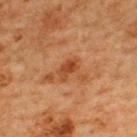Notes:
– workup — catalogued during a skin exam; not biopsied
– diameter — ≈3 mm
– illumination — cross-polarized
– patient — male, aged 63–67
– site — the upper back
– image — ~15 mm tile from a whole-body skin photo